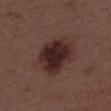biopsy status = no biopsy performed (imaged during a skin exam)
size = ~5 mm (longest diameter)
patient = male, aged 53–57
imaging modality = 15 mm crop, total-body photography
site = the upper back
lighting = white-light illumination
image-analysis metrics = border irregularity of about 1.5 on a 0–10 scale and internal color variation of about 4 on a 0–10 scale; a classifier nevus-likeness of about 95/100 and lesion-presence confidence of about 100/100